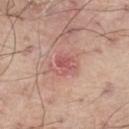Background: The patient is a male aged 68–72. A 15 mm close-up tile from a total-body photography series done for melanoma screening. The lesion is on the right thigh.